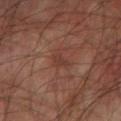The lesion was tiled from a total-body skin photograph and was not biopsied. Imaged with cross-polarized lighting. A male subject, aged 73 to 77. A region of skin cropped from a whole-body photographic capture, roughly 15 mm wide. The total-body-photography lesion software estimated an average lesion color of about L≈30 a*≈19 b*≈21 (CIELAB), about 5 CIELAB-L* units darker than the surrounding skin, and a normalized border contrast of about 5. The software also gave a border-irregularity rating of about 5/10 and a color-variation rating of about 0/10. On the arm. Approximately 2.5 mm at its widest.